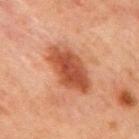• workup · total-body-photography surveillance lesion; no biopsy
• lighting · cross-polarized
• body site · the chest
• size · ≈6.5 mm
• image · 15 mm crop, total-body photography
• subject · male, about 65 years old